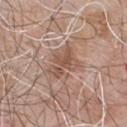Clinical impression: Recorded during total-body skin imaging; not selected for excision or biopsy. Context: Imaged with white-light lighting. The lesion is located on the chest. A region of skin cropped from a whole-body photographic capture, roughly 15 mm wide. The subject is a male about 65 years old. Longest diameter approximately 4 mm.